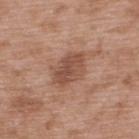{"biopsy_status": "not biopsied; imaged during a skin examination", "lesion_size": {"long_diameter_mm_approx": 4.5}, "patient": {"sex": "male", "age_approx": 50}, "site": "back", "lighting": "white-light", "image": {"source": "total-body photography crop", "field_of_view_mm": 15}}